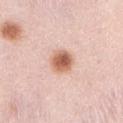• notes — no biopsy performed (imaged during a skin exam)
• lesion diameter — about 3 mm
• patient — female, aged approximately 40
• anatomic site — the abdomen
• image — ~15 mm crop, total-body skin-cancer survey
• automated metrics — a lesion area of about 7.5 mm², a shape eccentricity near 0.3, and two-axis asymmetry of about 0.15; a mean CIELAB color near L≈64 a*≈23 b*≈31, roughly 15 lightness units darker than nearby skin, and a normalized lesion–skin contrast near 9.5; border irregularity of about 1.5 on a 0–10 scale and a within-lesion color-variation index near 5.5/10